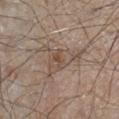Impression: Imaged during a routine full-body skin examination; the lesion was not biopsied and no histopathology is available. Image and clinical context: A 15 mm close-up tile from a total-body photography series done for melanoma screening. On the right lower leg. The subject is a male in their mid- to late 40s. Measured at roughly 6 mm in maximum diameter.